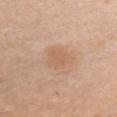| field | value |
|---|---|
| biopsy status | catalogued during a skin exam; not biopsied |
| patient | female, roughly 60 years of age |
| body site | the chest |
| lesion size | about 3.5 mm |
| image source | total-body-photography crop, ~15 mm field of view |